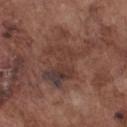Q: Who is the patient?
A: male, in their mid- to late 70s
Q: Where on the body is the lesion?
A: the chest
Q: How was the tile lit?
A: white-light illumination
Q: What did automated image analysis measure?
A: an average lesion color of about L≈37 a*≈18 b*≈22 (CIELAB) and a normalized border contrast of about 6.5; a border-irregularity index near 8.5/10, a color-variation rating of about 6.5/10, and a peripheral color-asymmetry measure near 1.5; an automated nevus-likeness rating near 0 out of 100 and lesion-presence confidence of about 80/100
Q: How was this image acquired?
A: 15 mm crop, total-body photography
Q: Lesion size?
A: ≈7.5 mm